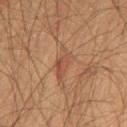No biopsy was performed on this lesion — it was imaged during a full skin examination and was not determined to be concerning. The total-body-photography lesion software estimated an average lesion color of about L≈41 a*≈19 b*≈27 (CIELAB) and a normalized lesion–skin contrast near 5. The analysis additionally found a border-irregularity index near 4/10, internal color variation of about 3.5 on a 0–10 scale, and peripheral color asymmetry of about 1. The software also gave a nevus-likeness score of about 0/100 and a lesion-detection confidence of about 95/100. On the left thigh. The lesion's longest dimension is about 4 mm. A 15 mm close-up extracted from a 3D total-body photography capture. The patient is a male aged 63–67. Captured under cross-polarized illumination.